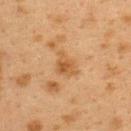biopsy status = no biopsy performed (imaged during a skin exam)
subject = female, aged approximately 40
location = the upper back
illumination = cross-polarized
imaging modality = total-body-photography crop, ~15 mm field of view
lesion size = about 3.5 mm